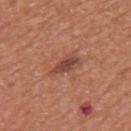This lesion was catalogued during total-body skin photography and was not selected for biopsy. An algorithmic analysis of the crop reported a lesion–skin lightness drop of about 11 and a normalized lesion–skin contrast near 8.5. The software also gave an automated nevus-likeness rating near 40 out of 100 and a lesion-detection confidence of about 100/100. A male subject, aged around 65. From the mid back. Measured at roughly 3.5 mm in maximum diameter. This image is a 15 mm lesion crop taken from a total-body photograph.